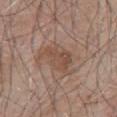Impression: No biopsy was performed on this lesion — it was imaged during a full skin examination and was not determined to be concerning. Acquisition and patient details: Located on the chest. The recorded lesion diameter is about 5 mm. A 15 mm crop from a total-body photograph taken for skin-cancer surveillance. Captured under white-light illumination. The patient is a male aged approximately 80.